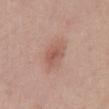The lesion was tiled from a total-body skin photograph and was not biopsied. A male subject, approximately 60 years of age. The lesion-visualizer software estimated a lesion area of about 5.5 mm², an outline eccentricity of about 0.75 (0 = round, 1 = elongated), and two-axis asymmetry of about 0.25. It also reported an average lesion color of about L≈55 a*≈23 b*≈27 (CIELAB), about 8 CIELAB-L* units darker than the surrounding skin, and a normalized lesion–skin contrast near 6. The software also gave border irregularity of about 3 on a 0–10 scale, a within-lesion color-variation index near 3/10, and radial color variation of about 1. The analysis additionally found a nevus-likeness score of about 80/100 and a lesion-detection confidence of about 100/100. Cropped from a whole-body photographic skin survey; the tile spans about 15 mm. The tile uses white-light illumination. From the front of the torso.